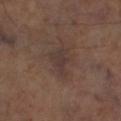Impression:
Part of a total-body skin-imaging series; this lesion was reviewed on a skin check and was not flagged for biopsy.
Image and clinical context:
The lesion is located on the left lower leg. Imaged with cross-polarized lighting. A roughly 15 mm field-of-view crop from a total-body skin photograph.